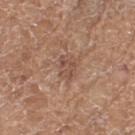<record>
<biopsy_status>not biopsied; imaged during a skin examination</biopsy_status>
<site>left thigh</site>
<lighting>white-light</lighting>
<automated_metrics>
  <vs_skin_contrast_norm>5.5</vs_skin_contrast_norm>
</automated_metrics>
<patient>
  <sex>female</sex>
  <age_approx>75</age_approx>
</patient>
<lesion_size>
  <long_diameter_mm_approx>3.0</long_diameter_mm_approx>
</lesion_size>
<image>
  <source>total-body photography crop</source>
  <field_of_view_mm>15</field_of_view_mm>
</image>
</record>